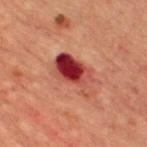Context: A 15 mm crop from a total-body photograph taken for skin-cancer surveillance. Measured at roughly 5.5 mm in maximum diameter. On the chest. The lesion-visualizer software estimated an area of roughly 13 mm², an eccentricity of roughly 0.85, and a symmetry-axis asymmetry near 0.35. And it measured a lesion–skin lightness drop of about 13 and a lesion-to-skin contrast of about 12 (normalized; higher = more distinct). It also reported a border-irregularity rating of about 4/10 and internal color variation of about 10 on a 0–10 scale. And it measured a classifier nevus-likeness of about 0/100. A male patient, roughly 60 years of age. Captured under cross-polarized illumination.